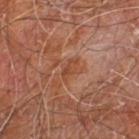{"biopsy_status": "not biopsied; imaged during a skin examination", "site": "right leg", "image": {"source": "total-body photography crop", "field_of_view_mm": 15}, "patient": {"sex": "male", "age_approx": 60}}